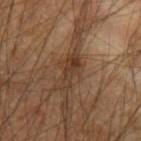Q: Was this lesion biopsied?
A: imaged on a skin check; not biopsied
Q: What kind of image is this?
A: ~15 mm crop, total-body skin-cancer survey
Q: What did automated image analysis measure?
A: a lesion color around L≈35 a*≈16 b*≈27 in CIELAB, a lesion–skin lightness drop of about 8, and a normalized border contrast of about 7.5; a border-irregularity index near 5/10, a within-lesion color-variation index near 3.5/10, and radial color variation of about 1
Q: Patient demographics?
A: male, aged around 65
Q: Lesion location?
A: the left forearm
Q: Illumination type?
A: cross-polarized illumination
Q: How large is the lesion?
A: ≈4 mm